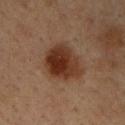| feature | finding |
|---|---|
| notes | no biopsy performed (imaged during a skin exam) |
| imaging modality | 15 mm crop, total-body photography |
| patient | male, aged 33–37 |
| automated lesion analysis | a mean CIELAB color near L≈30 a*≈19 b*≈27, roughly 12 lightness units darker than nearby skin, and a normalized lesion–skin contrast near 11.5; a within-lesion color-variation index near 5.5/10; a lesion-detection confidence of about 100/100 |
| size | ≈5.5 mm |
| body site | the upper back |